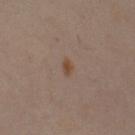Imaged during a routine full-body skin examination; the lesion was not biopsied and no histopathology is available. Captured under cross-polarized illumination. The total-body-photography lesion software estimated a symmetry-axis asymmetry near 0.35. The analysis additionally found a peripheral color-asymmetry measure near 0.5. The analysis additionally found an automated nevus-likeness rating near 85 out of 100 and lesion-presence confidence of about 100/100. The recorded lesion diameter is about 2 mm. The lesion is on the right upper arm. The subject is a female aged approximately 40. Cropped from a whole-body photographic skin survey; the tile spans about 15 mm.